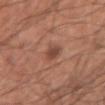Clinical impression:
The lesion was photographed on a routine skin check and not biopsied; there is no pathology result.
Context:
The lesion is on the right upper arm. The recorded lesion diameter is about 3 mm. This is a white-light tile. A region of skin cropped from a whole-body photographic capture, roughly 15 mm wide. Automated tile analysis of the lesion measured a border-irregularity rating of about 2.5/10, internal color variation of about 2.5 on a 0–10 scale, and a peripheral color-asymmetry measure near 1. The analysis additionally found a classifier nevus-likeness of about 85/100 and a lesion-detection confidence of about 100/100. A male subject, aged 58–62.